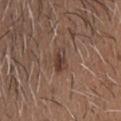• imaging modality · total-body-photography crop, ~15 mm field of view
• anatomic site · the head or neck
• subject · male, aged 48–52